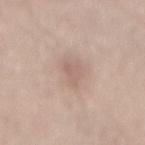Q: Was this lesion biopsied?
A: catalogued during a skin exam; not biopsied
Q: Automated lesion metrics?
A: a lesion area of about 4 mm² and two-axis asymmetry of about 0.45
Q: Patient demographics?
A: male, aged 43 to 47
Q: What is the lesion's diameter?
A: ~3.5 mm (longest diameter)
Q: Illumination type?
A: white-light
Q: What is the imaging modality?
A: ~15 mm tile from a whole-body skin photo
Q: Lesion location?
A: the mid back